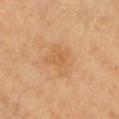Impression: Part of a total-body skin-imaging series; this lesion was reviewed on a skin check and was not flagged for biopsy. Acquisition and patient details: On the chest. The tile uses cross-polarized illumination. A region of skin cropped from a whole-body photographic capture, roughly 15 mm wide. A female patient, roughly 60 years of age. Longest diameter approximately 4 mm.